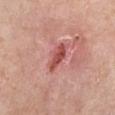Impression:
Captured during whole-body skin photography for melanoma surveillance; the lesion was not biopsied.
Context:
The patient is a female aged approximately 70. The lesion is located on the leg. A lesion tile, about 15 mm wide, cut from a 3D total-body photograph.